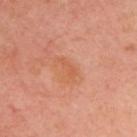Q: Was this lesion biopsied?
A: imaged on a skin check; not biopsied
Q: What kind of image is this?
A: ~15 mm tile from a whole-body skin photo
Q: How large is the lesion?
A: about 3 mm
Q: Lesion location?
A: the upper back
Q: What are the patient's age and sex?
A: male, aged around 50
Q: Automated lesion metrics?
A: a footprint of about 2.5 mm², a shape eccentricity near 0.95, and a shape-asymmetry score of about 0.45 (0 = symmetric)